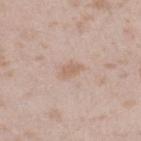{"biopsy_status": "not biopsied; imaged during a skin examination", "lighting": "white-light", "patient": {"sex": "female", "age_approx": 25}, "site": "right lower leg", "image": {"source": "total-body photography crop", "field_of_view_mm": 15}, "automated_metrics": {"area_mm2_approx": 3.0, "shape_asymmetry": 0.35}, "lesion_size": {"long_diameter_mm_approx": 2.5}}